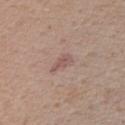Case summary:
- biopsy status · total-body-photography surveillance lesion; no biopsy
- size · ≈3 mm
- subject · male, aged 68 to 72
- body site · the chest
- acquisition · total-body-photography crop, ~15 mm field of view